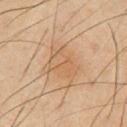The lesion was tiled from a total-body skin photograph and was not biopsied. The tile uses cross-polarized illumination. Automated tile analysis of the lesion measured a mean CIELAB color near L≈55 a*≈16 b*≈32, a lesion–skin lightness drop of about 6, and a normalized lesion–skin contrast near 5. A 15 mm close-up tile from a total-body photography series done for melanoma screening. The subject is a male aged around 40. Longest diameter approximately 5.5 mm. From the chest.